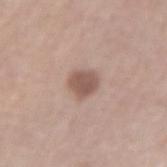No biopsy was performed on this lesion — it was imaged during a full skin examination and was not determined to be concerning. Approximately 3 mm at its widest. From the right forearm. A lesion tile, about 15 mm wide, cut from a 3D total-body photograph. A male patient, approximately 60 years of age. Automated image analysis of the tile measured a border-irregularity rating of about 2/10 and radial color variation of about 0.5. The analysis additionally found a classifier nevus-likeness of about 55/100.